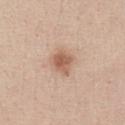| field | value |
|---|---|
| workup | total-body-photography surveillance lesion; no biopsy |
| lighting | white-light |
| diameter | ~3 mm (longest diameter) |
| image-analysis metrics | a shape eccentricity near 0.4 and a symmetry-axis asymmetry near 0.25; about 11 CIELAB-L* units darker than the surrounding skin and a normalized lesion–skin contrast near 7.5; border irregularity of about 2.5 on a 0–10 scale and peripheral color asymmetry of about 1 |
| imaging modality | 15 mm crop, total-body photography |
| patient | female, approximately 40 years of age |
| body site | the abdomen |